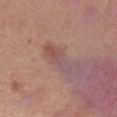| field | value |
|---|---|
| notes | total-body-photography surveillance lesion; no biopsy |
| TBP lesion metrics | an area of roughly 8 mm² and two-axis asymmetry of about 0.3; border irregularity of about 3.5 on a 0–10 scale and internal color variation of about 7.5 on a 0–10 scale |
| patient | female, aged approximately 35 |
| anatomic site | the right forearm |
| lesion size | ~5 mm (longest diameter) |
| image source | ~15 mm crop, total-body skin-cancer survey |